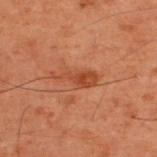| feature | finding |
|---|---|
| biopsy status | catalogued during a skin exam; not biopsied |
| patient | male, approximately 60 years of age |
| illumination | cross-polarized illumination |
| automated lesion analysis | an area of roughly 6 mm², a shape eccentricity near 0.95, and two-axis asymmetry of about 0.35; roughly 7 lightness units darker than nearby skin |
| site | the upper back |
| image source | total-body-photography crop, ~15 mm field of view |
| diameter | ~5 mm (longest diameter) |